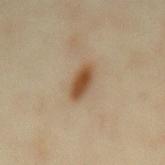- subject — female, in their mid-30s
- location — the mid back
- diameter — ≈4 mm
- lighting — cross-polarized
- image source — total-body-photography crop, ~15 mm field of view